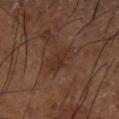| feature | finding |
|---|---|
| follow-up | catalogued during a skin exam; not biopsied |
| tile lighting | cross-polarized |
| lesion size | ~5 mm (longest diameter) |
| acquisition | 15 mm crop, total-body photography |
| automated lesion analysis | a lesion color around L≈31 a*≈19 b*≈26 in CIELAB, a lesion–skin lightness drop of about 6, and a lesion-to-skin contrast of about 6 (normalized; higher = more distinct); internal color variation of about 2 on a 0–10 scale and radial color variation of about 0.5 |
| site | the left lower leg |
| subject | male, approximately 65 years of age |